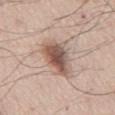imaging modality: ~15 mm tile from a whole-body skin photo; patient: male, in their mid- to late 50s; site: the chest.